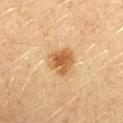Imaged during a routine full-body skin examination; the lesion was not biopsied and no histopathology is available. A roughly 15 mm field-of-view crop from a total-body skin photograph. Imaged with cross-polarized lighting. Measured at roughly 3.5 mm in maximum diameter. The total-body-photography lesion software estimated a classifier nevus-likeness of about 95/100 and lesion-presence confidence of about 100/100. The patient is a female aged around 20. Located on the left forearm.